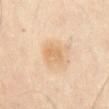Q: What lighting was used for the tile?
A: cross-polarized
Q: What is the lesion's diameter?
A: about 2.5 mm
Q: What did automated image analysis measure?
A: a classifier nevus-likeness of about 20/100 and lesion-presence confidence of about 100/100
Q: How was this image acquired?
A: ~15 mm crop, total-body skin-cancer survey
Q: Lesion location?
A: the abdomen
Q: Who is the patient?
A: male, aged 63–67